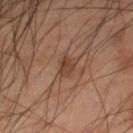Q: Was a biopsy performed?
A: total-body-photography surveillance lesion; no biopsy